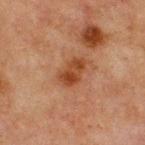biopsy status: catalogued during a skin exam; not biopsied | site: the chest | illumination: cross-polarized illumination | image source: 15 mm crop, total-body photography | patient: male, aged 63 to 67 | automated lesion analysis: border irregularity of about 2.5 on a 0–10 scale, a within-lesion color-variation index near 4/10, and radial color variation of about 1.5 | lesion diameter: ~3.5 mm (longest diameter).